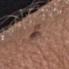| feature | finding |
|---|---|
| workup | total-body-photography surveillance lesion; no biopsy |
| tile lighting | white-light illumination |
| size | ≈2.5 mm |
| image | total-body-photography crop, ~15 mm field of view |
| anatomic site | the left forearm |
| subject | female, in their mid- to late 50s |